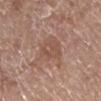Q: Was a biopsy performed?
A: no biopsy performed (imaged during a skin exam)
Q: What did automated image analysis measure?
A: border irregularity of about 4.5 on a 0–10 scale, a color-variation rating of about 3.5/10, and peripheral color asymmetry of about 1.5; a classifier nevus-likeness of about 0/100
Q: How was the tile lit?
A: white-light illumination
Q: Lesion size?
A: ~4 mm (longest diameter)
Q: What are the patient's age and sex?
A: male, aged approximately 70
Q: Lesion location?
A: the right lower leg
Q: What is the imaging modality?
A: 15 mm crop, total-body photography